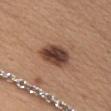workup: no biopsy performed (imaged during a skin exam).